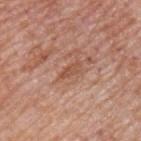• biopsy status · no biopsy performed (imaged during a skin exam)
• TBP lesion metrics · an area of roughly 2.5 mm², an outline eccentricity of about 0.9 (0 = round, 1 = elongated), and a shape-asymmetry score of about 0.4 (0 = symmetric); a lesion color around L≈52 a*≈23 b*≈31 in CIELAB and about 8 CIELAB-L* units darker than the surrounding skin; a border-irregularity index near 4/10 and a peripheral color-asymmetry measure near 0; an automated nevus-likeness rating near 0 out of 100 and a detector confidence of about 80 out of 100 that the crop contains a lesion
• patient · male, aged 73–77
• tile lighting · white-light illumination
• image · 15 mm crop, total-body photography
• size · ~2.5 mm (longest diameter)
• site · the chest